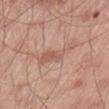Impression: The lesion was tiled from a total-body skin photograph and was not biopsied. Context: The patient is a male about 55 years old. The tile uses white-light illumination. Cropped from a whole-body photographic skin survey; the tile spans about 15 mm. Located on the leg.